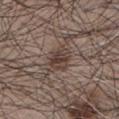{"biopsy_status": "not biopsied; imaged during a skin examination", "site": "chest", "patient": {"sex": "male", "age_approx": 65}, "lesion_size": {"long_diameter_mm_approx": 3.5}, "lighting": "white-light", "automated_metrics": {"cielab_L": 40, "cielab_a": 14, "cielab_b": 21, "vs_skin_darker_L": 9.0, "vs_skin_contrast_norm": 7.5}, "image": {"source": "total-body photography crop", "field_of_view_mm": 15}}